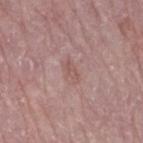Part of a total-body skin-imaging series; this lesion was reviewed on a skin check and was not flagged for biopsy.
A region of skin cropped from a whole-body photographic capture, roughly 15 mm wide.
Measured at roughly 3 mm in maximum diameter.
Imaged with white-light lighting.
The lesion is located on the right thigh.
A female patient, aged 63–67.
Automated image analysis of the tile measured an area of roughly 4 mm² and two-axis asymmetry of about 0.25. The software also gave a lesion color around L≈55 a*≈19 b*≈22 in CIELAB and about 6 CIELAB-L* units darker than the surrounding skin. The software also gave an automated nevus-likeness rating near 0 out of 100 and lesion-presence confidence of about 100/100.